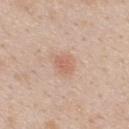Impression:
Recorded during total-body skin imaging; not selected for excision or biopsy.
Context:
Cropped from a total-body skin-imaging series; the visible field is about 15 mm. Measured at roughly 2.5 mm in maximum diameter. Located on the upper back. This is a white-light tile. A male patient in their mid-20s.